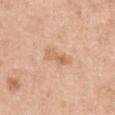• workup — imaged on a skin check; not biopsied
• lesion diameter — ~3.5 mm (longest diameter)
• TBP lesion metrics — a footprint of about 4.5 mm² and a shape-asymmetry score of about 0.25 (0 = symmetric); a lesion color around L≈65 a*≈21 b*≈35 in CIELAB, about 8 CIELAB-L* units darker than the surrounding skin, and a normalized lesion–skin contrast near 6; a classifier nevus-likeness of about 0/100
• location — the chest
• lighting — white-light illumination
• subject — female, aged approximately 40
• imaging modality — ~15 mm tile from a whole-body skin photo